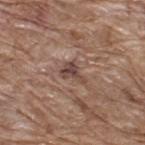Case summary:
• notes · catalogued during a skin exam; not biopsied
• anatomic site · the upper back
• image source · total-body-photography crop, ~15 mm field of view
• patient · male, aged 68 to 72